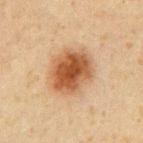Impression:
This lesion was catalogued during total-body skin photography and was not selected for biopsy.
Acquisition and patient details:
The lesion's longest dimension is about 5 mm. Located on the chest. A male patient aged 58–62. The total-body-photography lesion software estimated a lesion color around L≈45 a*≈20 b*≈32 in CIELAB, roughly 14 lightness units darker than nearby skin, and a normalized border contrast of about 10.5. The analysis additionally found a border-irregularity rating of about 1.5/10 and radial color variation of about 1.5. The analysis additionally found a classifier nevus-likeness of about 100/100 and lesion-presence confidence of about 100/100. This image is a 15 mm lesion crop taken from a total-body photograph.